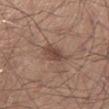<tbp_lesion>
<biopsy_status>not biopsied; imaged during a skin examination</biopsy_status>
<lesion_size>
  <long_diameter_mm_approx>4.5</long_diameter_mm_approx>
</lesion_size>
<automated_metrics>
  <area_mm2_approx>6.5</area_mm2_approx>
  <eccentricity>0.9</eccentricity>
  <shape_asymmetry>0.2</shape_asymmetry>
</automated_metrics>
<patient>
  <sex>male</sex>
  <age_approx>30</age_approx>
</patient>
<image>
  <source>total-body photography crop</source>
  <field_of_view_mm>15</field_of_view_mm>
</image>
<site>leg</site>
</tbp_lesion>